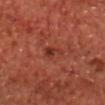The lesion was photographed on a routine skin check and not biopsied; there is no pathology result. The lesion is on the chest. A male subject about 70 years old. Automated tile analysis of the lesion measured a lesion color around L≈34 a*≈28 b*≈28 in CIELAB, roughly 8 lightness units darker than nearby skin, and a normalized lesion–skin contrast near 7. The analysis additionally found a border-irregularity index near 4.5/10, internal color variation of about 2 on a 0–10 scale, and a peripheral color-asymmetry measure near 0.5. The software also gave a classifier nevus-likeness of about 10/100 and a detector confidence of about 100 out of 100 that the crop contains a lesion. A 15 mm close-up extracted from a 3D total-body photography capture. The lesion's longest dimension is about 2.5 mm.